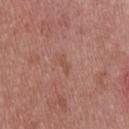follow-up: total-body-photography surveillance lesion; no biopsy
subject: male, aged 28–32
lighting: white-light
image: ~15 mm tile from a whole-body skin photo